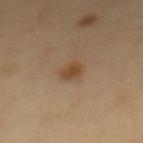Impression:
Part of a total-body skin-imaging series; this lesion was reviewed on a skin check and was not flagged for biopsy.
Background:
The tile uses cross-polarized illumination. On the mid back. A 15 mm close-up tile from a total-body photography series done for melanoma screening. A female patient, approximately 60 years of age.